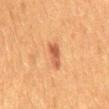Assessment:
Part of a total-body skin-imaging series; this lesion was reviewed on a skin check and was not flagged for biopsy.
Context:
This is a cross-polarized tile. Approximately 4 mm at its widest. A male patient, in their 50s. The lesion is on the mid back. A 15 mm close-up extracted from a 3D total-body photography capture.